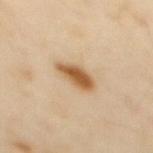notes=no biopsy performed (imaged during a skin exam)
site=the upper back
image-analysis metrics=a symmetry-axis asymmetry near 0.25; a mean CIELAB color near L≈59 a*≈19 b*≈39, a lesion–skin lightness drop of about 15, and a normalized lesion–skin contrast near 10.5; a classifier nevus-likeness of about 100/100 and lesion-presence confidence of about 100/100
diameter=~4 mm (longest diameter)
subject=female, aged around 45
tile lighting=cross-polarized
image source=15 mm crop, total-body photography